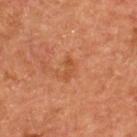workup=imaged on a skin check; not biopsied
imaging modality=~15 mm crop, total-body skin-cancer survey
location=the back
tile lighting=cross-polarized illumination
size=≈2.5 mm
subject=male, aged 53 to 57